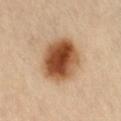Recorded during total-body skin imaging; not selected for excision or biopsy. Captured under cross-polarized illumination. A roughly 15 mm field-of-view crop from a total-body skin photograph. An algorithmic analysis of the crop reported a footprint of about 21 mm², a shape eccentricity near 0.65, and a symmetry-axis asymmetry near 0.1. It also reported radial color variation of about 3. The software also gave a detector confidence of about 100 out of 100 that the crop contains a lesion. From the abdomen. About 6 mm across.